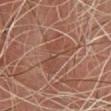Captured during whole-body skin photography for melanoma surveillance; the lesion was not biopsied. A lesion tile, about 15 mm wide, cut from a 3D total-body photograph. A male patient in their mid-40s. Imaged with cross-polarized lighting. On the chest.